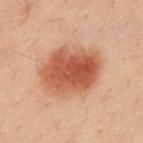biopsy status — catalogued during a skin exam; not biopsied